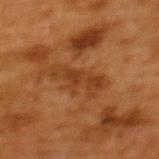This lesion was catalogued during total-body skin photography and was not selected for biopsy.